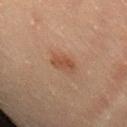Assessment: Part of a total-body skin-imaging series; this lesion was reviewed on a skin check and was not flagged for biopsy. Image and clinical context: The recorded lesion diameter is about 3 mm. The patient is a male approximately 30 years of age. A close-up tile cropped from a whole-body skin photograph, about 15 mm across. From the leg. Imaged with cross-polarized lighting.